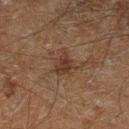Notes:
- follow-up: no biopsy performed (imaged during a skin exam)
- location: the leg
- diameter: about 3 mm
- acquisition: total-body-photography crop, ~15 mm field of view
- illumination: cross-polarized
- subject: male, in their 60s